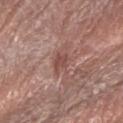Part of a total-body skin-imaging series; this lesion was reviewed on a skin check and was not flagged for biopsy. A 15 mm close-up tile from a total-body photography series done for melanoma screening. A female patient, roughly 75 years of age. The lesion is on the right forearm.